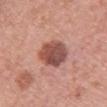{
  "biopsy_status": "not biopsied; imaged during a skin examination",
  "image": {
    "source": "total-body photography crop",
    "field_of_view_mm": 15
  },
  "lesion_size": {
    "long_diameter_mm_approx": 4.5
  },
  "lighting": "white-light",
  "patient": {
    "sex": "female",
    "age_approx": 60
  },
  "site": "chest"
}